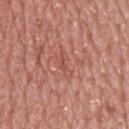{
  "biopsy_status": "not biopsied; imaged during a skin examination",
  "lesion_size": {
    "long_diameter_mm_approx": 3.5
  },
  "patient": {
    "sex": "male",
    "age_approx": 75
  },
  "site": "upper back",
  "automated_metrics": {
    "area_mm2_approx": 3.5,
    "eccentricity": 0.9,
    "shape_asymmetry": 0.6,
    "cielab_L": 52,
    "cielab_a": 27,
    "cielab_b": 29,
    "vs_skin_darker_L": 6.0,
    "vs_skin_contrast_norm": 4.5,
    "nevus_likeness_0_100": 0,
    "lesion_detection_confidence_0_100": 65
  },
  "lighting": "white-light",
  "image": {
    "source": "total-body photography crop",
    "field_of_view_mm": 15
  }
}